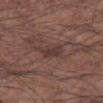Part of a total-body skin-imaging series; this lesion was reviewed on a skin check and was not flagged for biopsy.
The lesion's longest dimension is about 3 mm.
A 15 mm crop from a total-body photograph taken for skin-cancer surveillance.
This is a white-light tile.
The lesion is located on the right forearm.
The subject is a male in their mid- to late 50s.